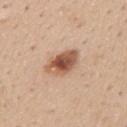Impression:
This lesion was catalogued during total-body skin photography and was not selected for biopsy.
Context:
The lesion is on the mid back. Cropped from a whole-body photographic skin survey; the tile spans about 15 mm. The patient is a male aged 28–32.